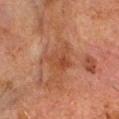Context:
This image is a 15 mm lesion crop taken from a total-body photograph. A male patient, approximately 80 years of age. From the head or neck. Longest diameter approximately 3 mm. Captured under cross-polarized illumination. An algorithmic analysis of the crop reported a mean CIELAB color near L≈36 a*≈21 b*≈28, a lesion–skin lightness drop of about 6, and a normalized border contrast of about 5.5. It also reported a border-irregularity index near 5/10, a color-variation rating of about 4/10, and a peripheral color-asymmetry measure near 1.5.